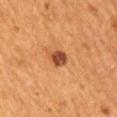The lesion was photographed on a routine skin check and not biopsied; there is no pathology result.
A male subject aged 53 to 57.
The lesion is on the right upper arm.
The recorded lesion diameter is about 2.5 mm.
Imaged with cross-polarized lighting.
Cropped from a total-body skin-imaging series; the visible field is about 15 mm.
Automated image analysis of the tile measured a footprint of about 4.5 mm², an outline eccentricity of about 0.65 (0 = round, 1 = elongated), and a shape-asymmetry score of about 0.2 (0 = symmetric). And it measured a nevus-likeness score of about 95/100 and a detector confidence of about 100 out of 100 that the crop contains a lesion.